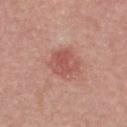Impression:
The lesion was photographed on a routine skin check and not biopsied; there is no pathology result.